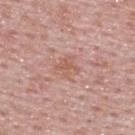Notes:
• follow-up — imaged on a skin check; not biopsied
• subject — male, about 50 years old
• image source — total-body-photography crop, ~15 mm field of view
• illumination — white-light
• site — the upper back
• lesion diameter — ≈3 mm